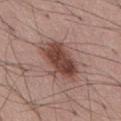lighting — white-light illumination | location — the abdomen | diameter — about 6 mm | automated metrics — an area of roughly 16 mm², an eccentricity of roughly 0.8, and two-axis asymmetry of about 0.2; an average lesion color of about L≈45 a*≈20 b*≈23 (CIELAB); a border-irregularity rating of about 3/10, internal color variation of about 5 on a 0–10 scale, and radial color variation of about 2; a classifier nevus-likeness of about 85/100 and lesion-presence confidence of about 100/100 | patient — male, aged 53 to 57 | acquisition — ~15 mm crop, total-body skin-cancer survey.